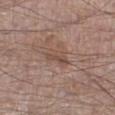| key | value |
|---|---|
| workup | total-body-photography surveillance lesion; no biopsy |
| imaging modality | ~15 mm tile from a whole-body skin photo |
| anatomic site | the right lower leg |
| patient | male, aged around 65 |